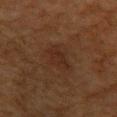<record>
  <biopsy_status>not biopsied; imaged during a skin examination</biopsy_status>
  <automated_metrics>
    <area_mm2_approx>6.5</area_mm2_approx>
    <eccentricity>0.7</eccentricity>
    <shape_asymmetry>0.25</shape_asymmetry>
    <lesion_detection_confidence_0_100>100</lesion_detection_confidence_0_100>
  </automated_metrics>
  <site>left upper arm</site>
  <image>
    <source>total-body photography crop</source>
    <field_of_view_mm>15</field_of_view_mm>
  </image>
  <patient>
    <sex>female</sex>
    <age_approx>55</age_approx>
  </patient>
  <lighting>cross-polarized</lighting>
</record>